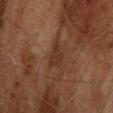{
  "biopsy_status": "not biopsied; imaged during a skin examination",
  "site": "upper back",
  "lesion_size": {
    "long_diameter_mm_approx": 3.0
  },
  "patient": {
    "sex": "male",
    "age_approx": 75
  },
  "lighting": "cross-polarized",
  "image": {
    "source": "total-body photography crop",
    "field_of_view_mm": 15
  }
}